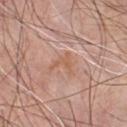Assessment: This lesion was catalogued during total-body skin photography and was not selected for biopsy. Clinical summary: The lesion is on the chest. The tile uses white-light illumination. A male subject, roughly 55 years of age. The lesion's longest dimension is about 3 mm. Cropped from a total-body skin-imaging series; the visible field is about 15 mm.